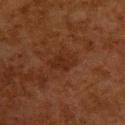Part of a total-body skin-imaging series; this lesion was reviewed on a skin check and was not flagged for biopsy.
On the upper back.
A region of skin cropped from a whole-body photographic capture, roughly 15 mm wide.
Measured at roughly 3.5 mm in maximum diameter.
A female subject, roughly 50 years of age.